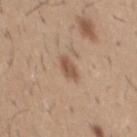Imaged during a routine full-body skin examination; the lesion was not biopsied and no histopathology is available.